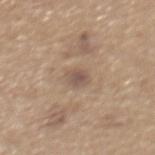Clinical impression: The lesion was photographed on a routine skin check and not biopsied; there is no pathology result. Acquisition and patient details: About 3 mm across. Captured under white-light illumination. This image is a 15 mm lesion crop taken from a total-body photograph. A male subject aged 63 to 67. The lesion-visualizer software estimated a shape eccentricity near 0.75. And it measured a lesion–skin lightness drop of about 9 and a normalized lesion–skin contrast near 7. And it measured a border-irregularity rating of about 1.5/10, a color-variation rating of about 2/10, and a peripheral color-asymmetry measure near 0.5. The analysis additionally found a nevus-likeness score of about 0/100 and lesion-presence confidence of about 100/100. On the mid back.